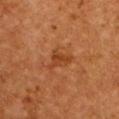Q: Was a biopsy performed?
A: no biopsy performed (imaged during a skin exam)
Q: What lighting was used for the tile?
A: cross-polarized
Q: What is the anatomic site?
A: the front of the torso
Q: What kind of image is this?
A: ~15 mm tile from a whole-body skin photo
Q: Who is the patient?
A: female, aged approximately 50
Q: Automated lesion metrics?
A: a lesion area of about 5.5 mm², an eccentricity of roughly 0.7, and a shape-asymmetry score of about 0.55 (0 = symmetric); a mean CIELAB color near L≈34 a*≈22 b*≈32, a lesion–skin lightness drop of about 6, and a lesion-to-skin contrast of about 6 (normalized; higher = more distinct)
Q: How large is the lesion?
A: ≈3.5 mm